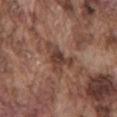Recorded during total-body skin imaging; not selected for excision or biopsy. Longest diameter approximately 4.5 mm. On the back. A 15 mm crop from a total-body photograph taken for skin-cancer surveillance. The subject is a male in their mid-70s. This is a white-light tile.